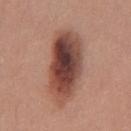This lesion was catalogued during total-body skin photography and was not selected for biopsy.
The patient is a male in their mid- to late 30s.
The lesion is on the abdomen.
A 15 mm crop from a total-body photograph taken for skin-cancer surveillance.